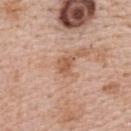Part of a total-body skin-imaging series; this lesion was reviewed on a skin check and was not flagged for biopsy. An algorithmic analysis of the crop reported a footprint of about 4 mm² and an eccentricity of roughly 0.7. The software also gave an average lesion color of about L≈57 a*≈22 b*≈32 (CIELAB) and a normalized border contrast of about 6.5. It also reported a border-irregularity rating of about 3.5/10 and peripheral color asymmetry of about 0.5. A close-up tile cropped from a whole-body skin photograph, about 15 mm across. On the back. Measured at roughly 2.5 mm in maximum diameter. This is a white-light tile. A female patient aged 48 to 52.